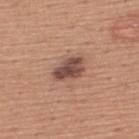{"biopsy_status": "not biopsied; imaged during a skin examination", "lighting": "white-light", "image": {"source": "total-body photography crop", "field_of_view_mm": 15}, "site": "upper back", "lesion_size": {"long_diameter_mm_approx": 4.0}, "patient": {"sex": "male", "age_approx": 45}}